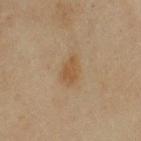patient: female, aged around 60; diameter: about 3.5 mm; illumination: cross-polarized illumination; anatomic site: the arm; image: total-body-photography crop, ~15 mm field of view.